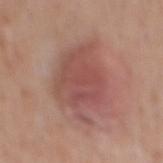Clinical impression: Imaged during a routine full-body skin examination; the lesion was not biopsied and no histopathology is available. Image and clinical context: A 15 mm close-up extracted from a 3D total-body photography capture. A male subject aged 63 to 67. Captured under white-light illumination. Longest diameter approximately 7.5 mm. The lesion is located on the mid back.